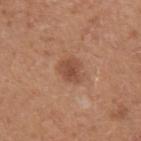Q: What is the lesion's diameter?
A: ~3 mm (longest diameter)
Q: Illumination type?
A: white-light illumination
Q: Automated lesion metrics?
A: an outline eccentricity of about 0.5 (0 = round, 1 = elongated) and a symmetry-axis asymmetry near 0.3; about 9 CIELAB-L* units darker than the surrounding skin and a normalized border contrast of about 7; a border-irregularity rating of about 2.5/10, internal color variation of about 2.5 on a 0–10 scale, and peripheral color asymmetry of about 1
Q: How was this image acquired?
A: total-body-photography crop, ~15 mm field of view
Q: Where on the body is the lesion?
A: the left upper arm
Q: What are the patient's age and sex?
A: male, about 40 years old